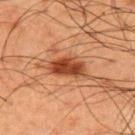{"biopsy_status": "not biopsied; imaged during a skin examination", "lighting": "cross-polarized", "patient": {"sex": "male", "age_approx": 60}, "lesion_size": {"long_diameter_mm_approx": 5.0}, "site": "upper back", "automated_metrics": {"nevus_likeness_0_100": 95, "lesion_detection_confidence_0_100": 100}, "image": {"source": "total-body photography crop", "field_of_view_mm": 15}}